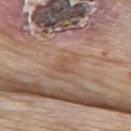Captured during whole-body skin photography for melanoma surveillance; the lesion was not biopsied. The lesion is located on the upper back. A male subject, aged approximately 85 or older. A lesion tile, about 15 mm wide, cut from a 3D total-body photograph.